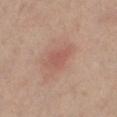{
  "biopsy_status": "not biopsied; imaged during a skin examination",
  "patient": {
    "sex": "female",
    "age_approx": 50
  },
  "image": {
    "source": "total-body photography crop",
    "field_of_view_mm": 15
  },
  "lighting": "cross-polarized",
  "site": "leg"
}